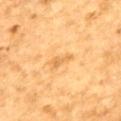Assessment: The lesion was photographed on a routine skin check and not biopsied; there is no pathology result. Acquisition and patient details: A 15 mm crop from a total-body photograph taken for skin-cancer surveillance. The subject is a male approximately 85 years of age or older. From the mid back. An algorithmic analysis of the crop reported an area of roughly 3 mm² and a shape-asymmetry score of about 0.45 (0 = symmetric). The tile uses cross-polarized illumination. Measured at roughly 3 mm in maximum diameter.